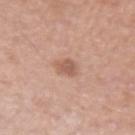workup: catalogued during a skin exam; not biopsied | image: ~15 mm tile from a whole-body skin photo | body site: the left upper arm | subject: male, about 40 years old.